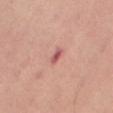Clinical impression: The lesion was tiled from a total-body skin photograph and was not biopsied. Background: An algorithmic analysis of the crop reported a lesion area of about 2.5 mm², an outline eccentricity of about 0.9 (0 = round, 1 = elongated), and a symmetry-axis asymmetry near 0.4. The analysis additionally found a classifier nevus-likeness of about 0/100 and lesion-presence confidence of about 100/100. A female patient, aged 38 to 42. A roughly 15 mm field-of-view crop from a total-body skin photograph. About 2.5 mm across. The lesion is on the right thigh.